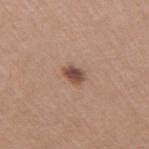Impression: Recorded during total-body skin imaging; not selected for excision or biopsy. Acquisition and patient details: On the right upper arm. An algorithmic analysis of the crop reported a mean CIELAB color near L≈48 a*≈20 b*≈27 and a lesion–skin lightness drop of about 14. And it measured a within-lesion color-variation index near 3/10 and radial color variation of about 1. The recorded lesion diameter is about 2.5 mm. A lesion tile, about 15 mm wide, cut from a 3D total-body photograph. A male patient, about 40 years old.